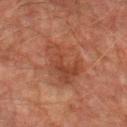No biopsy was performed on this lesion — it was imaged during a full skin examination and was not determined to be concerning.